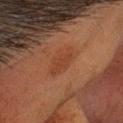Case summary:
- notes · no biopsy performed (imaged during a skin exam)
- automated lesion analysis · an average lesion color of about L≈39 a*≈24 b*≈31 (CIELAB), about 5 CIELAB-L* units darker than the surrounding skin, and a normalized lesion–skin contrast near 5; a border-irregularity rating of about 2/10, internal color variation of about 2 on a 0–10 scale, and a peripheral color-asymmetry measure near 0.5
- subject · male, aged 58–62
- anatomic site · the head or neck
- illumination · cross-polarized
- acquisition · 15 mm crop, total-body photography